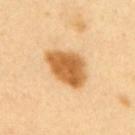{"patient": {"sex": "male", "age_approx": 40}, "automated_metrics": {"area_mm2_approx": 16.0, "eccentricity": 0.75, "shape_asymmetry": 0.2}, "image": {"source": "total-body photography crop", "field_of_view_mm": 15}, "site": "upper back", "lesion_size": {"long_diameter_mm_approx": 5.5}}